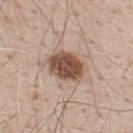Assessment: The lesion was tiled from a total-body skin photograph and was not biopsied. Clinical summary: From the upper back. The recorded lesion diameter is about 4.5 mm. A male patient, roughly 70 years of age. Cropped from a whole-body photographic skin survey; the tile spans about 15 mm.